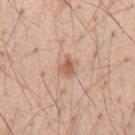Findings:
* anatomic site — the mid back
* patient — male, aged 53 to 57
* acquisition — ~15 mm tile from a whole-body skin photo
* lesion diameter — about 2.5 mm
* lighting — white-light